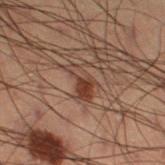Q: Was this lesion biopsied?
A: catalogued during a skin exam; not biopsied
Q: What lighting was used for the tile?
A: cross-polarized illumination
Q: How was this image acquired?
A: ~15 mm tile from a whole-body skin photo
Q: What is the lesion's diameter?
A: ~4 mm (longest diameter)
Q: Where on the body is the lesion?
A: the right thigh
Q: Who is the patient?
A: male, aged 53 to 57
Q: What did automated image analysis measure?
A: a lesion area of about 6 mm², an eccentricity of roughly 0.85, and a shape-asymmetry score of about 0.4 (0 = symmetric); roughly 8 lightness units darker than nearby skin